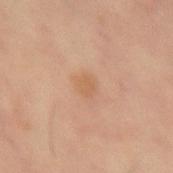This lesion was catalogued during total-body skin photography and was not selected for biopsy.
A 15 mm crop from a total-body photograph taken for skin-cancer surveillance.
The tile uses cross-polarized illumination.
The lesion is on the leg.
Approximately 2.5 mm at its widest.
The lesion-visualizer software estimated a mean CIELAB color near L≈56 a*≈19 b*≈32 and a normalized lesion–skin contrast near 4.5. It also reported an automated nevus-likeness rating near 0 out of 100 and lesion-presence confidence of about 100/100.
A male subject about 60 years old.